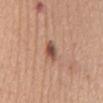Captured during whole-body skin photography for melanoma surveillance; the lesion was not biopsied.
Located on the mid back.
A region of skin cropped from a whole-body photographic capture, roughly 15 mm wide.
A female subject, aged 48 to 52.
The tile uses white-light illumination.
About 3 mm across.